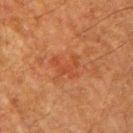Findings:
– biopsy status: imaged on a skin check; not biopsied
– location: the right upper arm
– illumination: cross-polarized
– TBP lesion metrics: an outline eccentricity of about 0.55 (0 = round, 1 = elongated) and two-axis asymmetry of about 0.6
– image source: total-body-photography crop, ~15 mm field of view
– subject: male, about 80 years old
– lesion size: about 3 mm